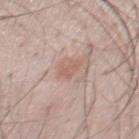biopsy status = imaged on a skin check; not biopsied | diameter = ≈2.5 mm | location = the chest | subject = male, about 50 years old | image source = total-body-photography crop, ~15 mm field of view.